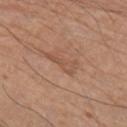This lesion was catalogued during total-body skin photography and was not selected for biopsy.
A male patient aged 78–82.
Automated tile analysis of the lesion measured an area of roughly 4.5 mm², an eccentricity of roughly 0.95, and two-axis asymmetry of about 0.55.
On the right upper arm.
Captured under white-light illumination.
The recorded lesion diameter is about 4 mm.
A 15 mm close-up extracted from a 3D total-body photography capture.